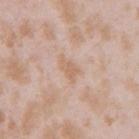Imaged during a routine full-body skin examination; the lesion was not biopsied and no histopathology is available.
An algorithmic analysis of the crop reported a lesion area of about 3.5 mm² and an outline eccentricity of about 0.8 (0 = round, 1 = elongated).
On the right upper arm.
A 15 mm close-up extracted from a 3D total-body photography capture.
Approximately 3 mm at its widest.
A female patient, roughly 25 years of age.